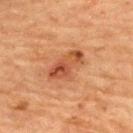The lesion was photographed on a routine skin check and not biopsied; there is no pathology result. About 5 mm across. A female patient aged approximately 70. Imaged with cross-polarized lighting. Located on the upper back. Cropped from a total-body skin-imaging series; the visible field is about 15 mm.